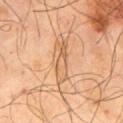<tbp_lesion>
  <biopsy_status>not biopsied; imaged during a skin examination</biopsy_status>
  <lesion_size>
    <long_diameter_mm_approx>5.0</long_diameter_mm_approx>
  </lesion_size>
  <image>
    <source>total-body photography crop</source>
    <field_of_view_mm>15</field_of_view_mm>
  </image>
  <patient>
    <sex>male</sex>
    <age_approx>65</age_approx>
  </patient>
  <site>arm</site>
</tbp_lesion>